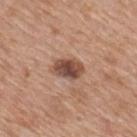Findings:
– workup — catalogued during a skin exam; not biopsied
– TBP lesion metrics — an automated nevus-likeness rating near 75 out of 100 and a detector confidence of about 100 out of 100 that the crop contains a lesion
– size — about 4 mm
– patient — male, approximately 60 years of age
– acquisition — total-body-photography crop, ~15 mm field of view
– anatomic site — the mid back
– lighting — white-light